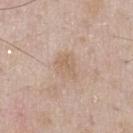Q: Was a biopsy performed?
A: total-body-photography surveillance lesion; no biopsy
Q: What are the patient's age and sex?
A: male, aged 53–57
Q: What is the lesion's diameter?
A: ~3 mm (longest diameter)
Q: What lighting was used for the tile?
A: white-light
Q: Where on the body is the lesion?
A: the chest
Q: What is the imaging modality?
A: ~15 mm crop, total-body skin-cancer survey